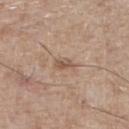Impression:
Part of a total-body skin-imaging series; this lesion was reviewed on a skin check and was not flagged for biopsy.
Background:
The patient is a male in their mid-60s. Cropped from a total-body skin-imaging series; the visible field is about 15 mm. The tile uses white-light illumination. Located on the right lower leg.